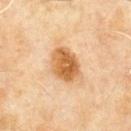{"biopsy_status": "not biopsied; imaged during a skin examination", "patient": {"sex": "male", "age_approx": 60}, "site": "mid back", "automated_metrics": {"border_irregularity_0_10": 1.5, "color_variation_0_10": 5.5, "peripheral_color_asymmetry": 2.0}, "image": {"source": "total-body photography crop", "field_of_view_mm": 15}, "lesion_size": {"long_diameter_mm_approx": 4.0}, "lighting": "cross-polarized"}